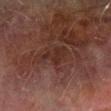– workup · no biopsy performed (imaged during a skin exam)
– subject · male, in their mid-70s
– site · the left forearm
– illumination · cross-polarized illumination
– lesion size · about 3 mm
– acquisition · 15 mm crop, total-body photography